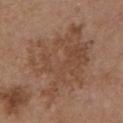The total-body-photography lesion software estimated an area of roughly 48 mm², a shape eccentricity near 0.6, and a symmetry-axis asymmetry near 0.35. And it measured an average lesion color of about L≈47 a*≈18 b*≈29 (CIELAB) and a normalized border contrast of about 5.5. The analysis additionally found a color-variation rating of about 4.5/10 and radial color variation of about 2. And it measured a classifier nevus-likeness of about 0/100. About 10.5 mm across. The tile uses white-light illumination. A 15 mm close-up extracted from a 3D total-body photography capture. The lesion is located on the chest. A female subject, approximately 65 years of age.